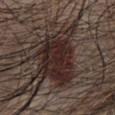{
  "biopsy_status": "not biopsied; imaged during a skin examination",
  "image": {
    "source": "total-body photography crop",
    "field_of_view_mm": 15
  },
  "patient": {
    "sex": "male",
    "age_approx": 50
  },
  "lighting": "white-light",
  "site": "chest"
}